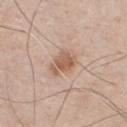follow-up=total-body-photography surveillance lesion; no biopsy
subject=male, about 45 years old
anatomic site=the chest
image source=15 mm crop, total-body photography